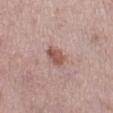  image:
    source: total-body photography crop
    field_of_view_mm: 15
  lesion_size:
    long_diameter_mm_approx: 2.5
  automated_metrics:
    area_mm2_approx: 5.0
    eccentricity: 0.5
    shape_asymmetry: 0.2
  patient:
    sex: female
    age_approx: 40
  lighting: white-light
  site: right lower leg